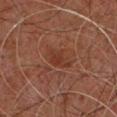notes: catalogued during a skin exam; not biopsied
subject: male, approximately 60 years of age
imaging modality: 15 mm crop, total-body photography
site: the upper back
lighting: cross-polarized illumination